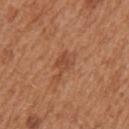workup = imaged on a skin check; not biopsied
lighting = white-light
diameter = ~4 mm (longest diameter)
image = ~15 mm tile from a whole-body skin photo
location = the left upper arm
subject = female, aged 38–42
automated lesion analysis = a footprint of about 5 mm² and two-axis asymmetry of about 0.5; an automated nevus-likeness rating near 0 out of 100 and a detector confidence of about 100 out of 100 that the crop contains a lesion